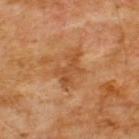Captured during whole-body skin photography for melanoma surveillance; the lesion was not biopsied.
A close-up tile cropped from a whole-body skin photograph, about 15 mm across.
The subject is a male aged approximately 60.
The lesion is located on the chest.